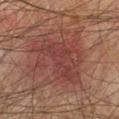Case summary:
- notes — imaged on a skin check; not biopsied
- image — 15 mm crop, total-body photography
- site — the left forearm
- subject — male, approximately 75 years of age
- diameter — about 7 mm
- image-analysis metrics — a border-irregularity index near 7/10, a within-lesion color-variation index near 3.5/10, and peripheral color asymmetry of about 1; a nevus-likeness score of about 0/100 and a detector confidence of about 100 out of 100 that the crop contains a lesion
- tile lighting — cross-polarized illumination